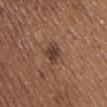Captured during whole-body skin photography for melanoma surveillance; the lesion was not biopsied. Cropped from a total-body skin-imaging series; the visible field is about 15 mm. Located on the upper back. The subject is a male about 65 years old.